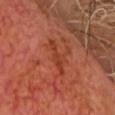Part of a total-body skin-imaging series; this lesion was reviewed on a skin check and was not flagged for biopsy. Measured at roughly 4 mm in maximum diameter. A male subject approximately 65 years of age. A 15 mm close-up extracted from a 3D total-body photography capture. Imaged with cross-polarized lighting.